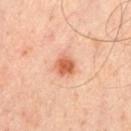workup — catalogued during a skin exam; not biopsied | subject — male, about 55 years old | acquisition — total-body-photography crop, ~15 mm field of view | location — the chest.